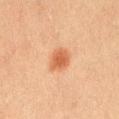This image is a 15 mm lesion crop taken from a total-body photograph. Captured under cross-polarized illumination. The subject is a female aged approximately 20. Longest diameter approximately 3 mm. On the right thigh.